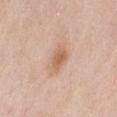notes — no biopsy performed (imaged during a skin exam); lesion diameter — ≈3.5 mm; body site — the back; imaging modality — total-body-photography crop, ~15 mm field of view; TBP lesion metrics — an average lesion color of about L≈63 a*≈20 b*≈31 (CIELAB) and a lesion–skin lightness drop of about 9; subject — female, about 60 years old; illumination — white-light.